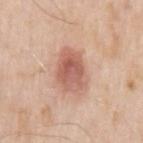Q: Was a biopsy performed?
A: imaged on a skin check; not biopsied
Q: What is the imaging modality?
A: ~15 mm tile from a whole-body skin photo
Q: Who is the patient?
A: male, aged approximately 55
Q: What lighting was used for the tile?
A: white-light
Q: Automated lesion metrics?
A: a lesion color around L≈60 a*≈24 b*≈28 in CIELAB, a lesion–skin lightness drop of about 12, and a normalized lesion–skin contrast near 7.5; a nevus-likeness score of about 90/100
Q: Where on the body is the lesion?
A: the arm